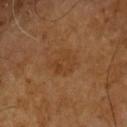<case>
<biopsy_status>not biopsied; imaged during a skin examination</biopsy_status>
<patient>
  <sex>male</sex>
  <age_approx>60</age_approx>
</patient>
<image>
  <source>total-body photography crop</source>
  <field_of_view_mm>15</field_of_view_mm>
</image>
<automated_metrics>
  <cielab_L>38</cielab_L>
  <cielab_a>20</cielab_a>
  <cielab_b>33</cielab_b>
  <vs_skin_darker_L>5.0</vs_skin_darker_L>
  <vs_skin_contrast_norm>5.0</vs_skin_contrast_norm>
  <border_irregularity_0_10>5.0</border_irregularity_0_10>
  <color_variation_0_10>2.5</color_variation_0_10>
  <peripheral_color_asymmetry>1.0</peripheral_color_asymmetry>
  <lesion_detection_confidence_0_100>100</lesion_detection_confidence_0_100>
</automated_metrics>
<lesion_size>
  <long_diameter_mm_approx>3.5</long_diameter_mm_approx>
</lesion_size>
<site>right arm</site>
</case>